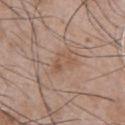biopsy status — catalogued during a skin exam; not biopsied | location — the chest | image-analysis metrics — an outline eccentricity of about 0.75 (0 = round, 1 = elongated) and a shape-asymmetry score of about 0.5 (0 = symmetric); a border-irregularity index near 4.5/10 and a color-variation rating of about 3.5/10; a classifier nevus-likeness of about 0/100 and lesion-presence confidence of about 100/100 | tile lighting — white-light | acquisition — ~15 mm crop, total-body skin-cancer survey | diameter — about 3.5 mm | patient — male, roughly 50 years of age.